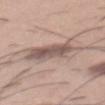workup: total-body-photography surveillance lesion; no biopsy | lighting: white-light illumination | TBP lesion metrics: an average lesion color of about L≈57 a*≈15 b*≈22 (CIELAB), about 10 CIELAB-L* units darker than the surrounding skin, and a lesion-to-skin contrast of about 7 (normalized; higher = more distinct); border irregularity of about 3.5 on a 0–10 scale, internal color variation of about 6 on a 0–10 scale, and peripheral color asymmetry of about 2; a classifier nevus-likeness of about 10/100 and a lesion-detection confidence of about 85/100 | image source: ~15 mm crop, total-body skin-cancer survey | site: the mid back | diameter: ≈5.5 mm | patient: male, in their 30s.